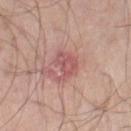biopsy_status: not biopsied; imaged during a skin examination
automated_metrics:
  area_mm2_approx: 7.0
  eccentricity: 0.75
  shape_asymmetry: 0.45
  cielab_L: 55
  cielab_a: 26
  cielab_b: 23
  vs_skin_darker_L: 9.0
  vs_skin_contrast_norm: 6.5
  nevus_likeness_0_100: 0
  lesion_detection_confidence_0_100: 100
lesion_size:
  long_diameter_mm_approx: 4.0
patient:
  sex: male
  age_approx: 70
site: right thigh
image:
  source: total-body photography crop
  field_of_view_mm: 15
lighting: white-light